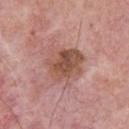Clinical impression:
The lesion was photographed on a routine skin check and not biopsied; there is no pathology result.
Clinical summary:
Located on the chest. The lesion's longest dimension is about 4.5 mm. Imaged with white-light lighting. The subject is a male in their mid- to late 70s. Automated tile analysis of the lesion measured an area of roughly 16 mm² and an eccentricity of roughly 0.3. And it measured a lesion color around L≈52 a*≈22 b*≈27 in CIELAB and roughly 10 lightness units darker than nearby skin. The analysis additionally found a border-irregularity rating of about 3.5/10, a color-variation rating of about 5.5/10, and radial color variation of about 2. A region of skin cropped from a whole-body photographic capture, roughly 15 mm wide.